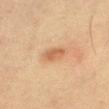* biopsy status — catalogued during a skin exam; not biopsied
* imaging modality — total-body-photography crop, ~15 mm field of view
* subject — female, aged approximately 50
* site — the right thigh
* TBP lesion metrics — a footprint of about 4 mm² and an outline eccentricity of about 0.8 (0 = round, 1 = elongated); a mean CIELAB color near L≈51 a*≈19 b*≈32 and a lesion–skin lightness drop of about 9; a color-variation rating of about 2/10 and radial color variation of about 1